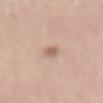illumination: white-light | subject: male, roughly 30 years of age | image source: ~15 mm tile from a whole-body skin photo | location: the back.